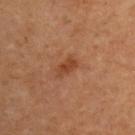No biopsy was performed on this lesion — it was imaged during a full skin examination and was not determined to be concerning. An algorithmic analysis of the crop reported a footprint of about 3 mm². The analysis additionally found a lesion color around L≈32 a*≈20 b*≈27 in CIELAB, roughly 7 lightness units darker than nearby skin, and a lesion-to-skin contrast of about 7 (normalized; higher = more distinct). The software also gave border irregularity of about 3 on a 0–10 scale, a within-lesion color-variation index near 1.5/10, and peripheral color asymmetry of about 0.5. A region of skin cropped from a whole-body photographic capture, roughly 15 mm wide. The lesion is located on the right upper arm. Imaged with cross-polarized lighting. The patient is a male aged approximately 70. Longest diameter approximately 2.5 mm.